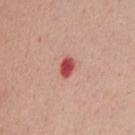biopsy_status: not biopsied; imaged during a skin examination
site: chest
image:
  source: total-body photography crop
  field_of_view_mm: 15
lighting: white-light
patient:
  sex: female
  age_approx: 40